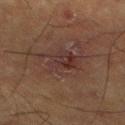Captured under cross-polarized illumination.
From the left lower leg.
A male subject, about 60 years old.
Measured at roughly 5.5 mm in maximum diameter.
A 15 mm close-up tile from a total-body photography series done for melanoma screening.